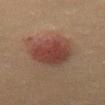biopsy_status: not biopsied; imaged during a skin examination
patient:
  sex: female
  age_approx: 40
image:
  source: total-body photography crop
  field_of_view_mm: 15
lighting: cross-polarized
site: left thigh
lesion_size:
  long_diameter_mm_approx: 5.5
automated_metrics:
  area_mm2_approx: 20.0